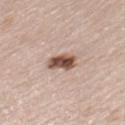No biopsy was performed on this lesion — it was imaged during a full skin examination and was not determined to be concerning. An algorithmic analysis of the crop reported a footprint of about 6 mm², an outline eccentricity of about 0.75 (0 = round, 1 = elongated), and a symmetry-axis asymmetry near 0.2. The software also gave a border-irregularity index near 2/10, a within-lesion color-variation index near 5.5/10, and radial color variation of about 2. About 3.5 mm across. A male patient, roughly 75 years of age. A 15 mm crop from a total-body photograph taken for skin-cancer surveillance. On the left thigh. Imaged with white-light lighting.